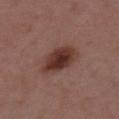Q: Is there a histopathology result?
A: imaged on a skin check; not biopsied
Q: Who is the patient?
A: female, approximately 30 years of age
Q: What is the anatomic site?
A: the upper back
Q: What did automated image analysis measure?
A: a lesion area of about 11 mm², an eccentricity of roughly 0.85, and a shape-asymmetry score of about 0.15 (0 = symmetric); an automated nevus-likeness rating near 100 out of 100 and lesion-presence confidence of about 100/100
Q: What is the imaging modality?
A: ~15 mm crop, total-body skin-cancer survey
Q: What is the lesion's diameter?
A: about 5 mm
Q: How was the tile lit?
A: white-light